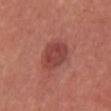Recorded during total-body skin imaging; not selected for excision or biopsy.
This is a white-light tile.
A region of skin cropped from a whole-body photographic capture, roughly 15 mm wide.
A female patient aged 33–37.
The lesion is located on the upper back.